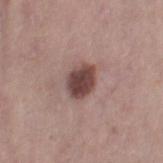Captured during whole-body skin photography for melanoma surveillance; the lesion was not biopsied.
A 15 mm close-up extracted from a 3D total-body photography capture.
About 3.5 mm across.
A female subject, aged 38–42.
The lesion is on the right thigh.
The tile uses white-light illumination.